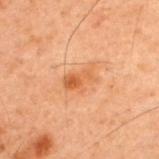{
  "biopsy_status": "not biopsied; imaged during a skin examination",
  "site": "upper back",
  "patient": {
    "sex": "male",
    "age_approx": 60
  },
  "lesion_size": {
    "long_diameter_mm_approx": 3.5
  },
  "image": {
    "source": "total-body photography crop",
    "field_of_view_mm": 15
  },
  "lighting": "cross-polarized"
}